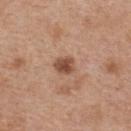On the back.
This is a white-light tile.
A roughly 15 mm field-of-view crop from a total-body skin photograph.
The lesion's longest dimension is about 2.5 mm.
The subject is a female aged around 40.